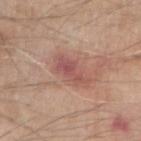follow-up: no biopsy performed (imaged during a skin exam)
site: the right upper arm
subject: male, in their mid-60s
imaging modality: ~15 mm crop, total-body skin-cancer survey
automated lesion analysis: a footprint of about 6.5 mm², an eccentricity of roughly 0.85, and a shape-asymmetry score of about 0.35 (0 = symmetric); border irregularity of about 5 on a 0–10 scale, a within-lesion color-variation index near 4/10, and a peripheral color-asymmetry measure near 1.5; a classifier nevus-likeness of about 0/100 and a lesion-detection confidence of about 100/100
diameter: about 4.5 mm